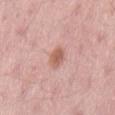{"biopsy_status": "not biopsied; imaged during a skin examination", "lighting": "white-light", "patient": {"sex": "male", "age_approx": 55}, "image": {"source": "total-body photography crop", "field_of_view_mm": 15}, "lesion_size": {"long_diameter_mm_approx": 2.5}, "site": "lower back"}